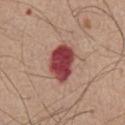No biopsy was performed on this lesion — it was imaged during a full skin examination and was not determined to be concerning.
A 15 mm close-up extracted from a 3D total-body photography capture.
The recorded lesion diameter is about 5 mm.
The subject is a male about 75 years old.
The tile uses white-light illumination.
On the abdomen.